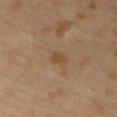This lesion was catalogued during total-body skin photography and was not selected for biopsy.
A male subject approximately 65 years of age.
Cropped from a total-body skin-imaging series; the visible field is about 15 mm.
This is a cross-polarized tile.